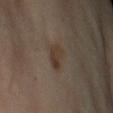Clinical impression: Recorded during total-body skin imaging; not selected for excision or biopsy. Context: Located on the left upper arm. A female subject approximately 55 years of age. A lesion tile, about 15 mm wide, cut from a 3D total-body photograph.